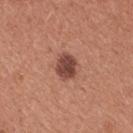Q: Is there a histopathology result?
A: no biopsy performed (imaged during a skin exam)
Q: What kind of image is this?
A: ~15 mm tile from a whole-body skin photo
Q: What is the anatomic site?
A: the chest
Q: Who is the patient?
A: female, aged 33–37